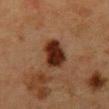notes = no biopsy performed (imaged during a skin exam).